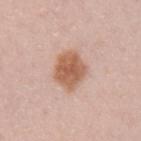Imaged during a routine full-body skin examination; the lesion was not biopsied and no histopathology is available. On the left upper arm. A region of skin cropped from a whole-body photographic capture, roughly 15 mm wide. The tile uses white-light illumination. A female subject, aged around 40. Measured at roughly 4.5 mm in maximum diameter.